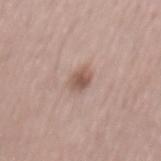{"biopsy_status": "not biopsied; imaged during a skin examination", "automated_metrics": {"cielab_L": 55, "cielab_a": 18, "cielab_b": 24, "vs_skin_darker_L": 11.0, "vs_skin_contrast_norm": 7.5, "color_variation_0_10": 3.0, "peripheral_color_asymmetry": 1.0, "nevus_likeness_0_100": 55, "lesion_detection_confidence_0_100": 100}, "image": {"source": "total-body photography crop", "field_of_view_mm": 15}, "patient": {"sex": "male", "age_approx": 55}, "lighting": "white-light"}